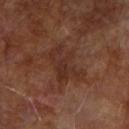body site: the right upper arm | subject: male, aged 63 to 67 | acquisition: ~15 mm crop, total-body skin-cancer survey.